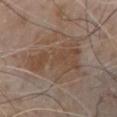The lesion was tiled from a total-body skin photograph and was not biopsied.
A roughly 15 mm field-of-view crop from a total-body skin photograph.
Measured at roughly 7 mm in maximum diameter.
Captured under white-light illumination.
Located on the chest.
A male subject aged around 80.